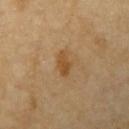follow-up: imaged on a skin check; not biopsied | acquisition: 15 mm crop, total-body photography | subject: female, aged 68–72 | size: ~3 mm (longest diameter) | automated metrics: about 9 CIELAB-L* units darker than the surrounding skin; a border-irregularity index near 2.5/10, a color-variation rating of about 2.5/10, and a peripheral color-asymmetry measure near 1; lesion-presence confidence of about 100/100 | body site: the left upper arm.